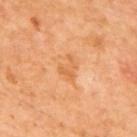Imaged during a routine full-body skin examination; the lesion was not biopsied and no histopathology is available. The tile uses cross-polarized illumination. A male subject, aged 63 to 67. From the upper back. A 15 mm crop from a total-body photograph taken for skin-cancer surveillance. The recorded lesion diameter is about 3 mm.